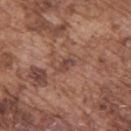| field | value |
|---|---|
| notes | imaged on a skin check; not biopsied |
| image | total-body-photography crop, ~15 mm field of view |
| lighting | white-light illumination |
| location | the back |
| subject | male, aged around 75 |
| automated metrics | an outline eccentricity of about 0.85 (0 = round, 1 = elongated) and two-axis asymmetry of about 0.35; a mean CIELAB color near L≈45 a*≈21 b*≈25; a nevus-likeness score of about 5/100 and a detector confidence of about 95 out of 100 that the crop contains a lesion |
| lesion diameter | ≈2.5 mm |